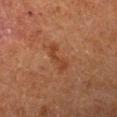The lesion was tiled from a total-body skin photograph and was not biopsied.
The lesion is on the arm.
Cropped from a whole-body photographic skin survey; the tile spans about 15 mm.
A female subject in their 50s.